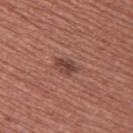From the upper back. Captured under white-light illumination. Measured at roughly 3 mm in maximum diameter. The subject is a female roughly 50 years of age. A region of skin cropped from a whole-body photographic capture, roughly 15 mm wide.